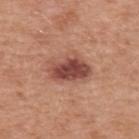{"biopsy_status": "not biopsied; imaged during a skin examination", "patient": {"sex": "male", "age_approx": 50}, "site": "upper back", "image": {"source": "total-body photography crop", "field_of_view_mm": 15}, "lighting": "white-light", "automated_metrics": {"area_mm2_approx": 13.0, "shape_asymmetry": 0.25, "cielab_L": 48, "cielab_a": 25, "cielab_b": 27, "vs_skin_darker_L": 13.0, "vs_skin_contrast_norm": 9.5, "border_irregularity_0_10": 3.0, "color_variation_0_10": 6.0, "peripheral_color_asymmetry": 2.0}}